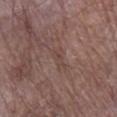No biopsy was performed on this lesion — it was imaged during a full skin examination and was not determined to be concerning. Imaged with white-light lighting. From the right lower leg. A lesion tile, about 15 mm wide, cut from a 3D total-body photograph. The recorded lesion diameter is about 3 mm. A male patient roughly 80 years of age.